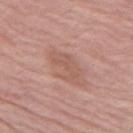| field | value |
|---|---|
| follow-up | catalogued during a skin exam; not biopsied |
| patient | female, aged 68 to 72 |
| body site | the left thigh |
| acquisition | total-body-photography crop, ~15 mm field of view |
| size | about 5 mm |
| tile lighting | white-light |